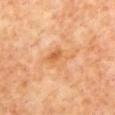<lesion>
  <biopsy_status>not biopsied; imaged during a skin examination</biopsy_status>
  <automated_metrics>
    <border_irregularity_0_10>4.0</border_irregularity_0_10>
    <color_variation_0_10>6.5</color_variation_0_10>
    <peripheral_color_asymmetry>2.0</peripheral_color_asymmetry>
    <nevus_likeness_0_100>0</nevus_likeness_0_100>
    <lesion_detection_confidence_0_100>100</lesion_detection_confidence_0_100>
  </automated_metrics>
  <image>
    <source>total-body photography crop</source>
    <field_of_view_mm>15</field_of_view_mm>
  </image>
  <lighting>cross-polarized</lighting>
  <lesion_size>
    <long_diameter_mm_approx>4.5</long_diameter_mm_approx>
  </lesion_size>
  <site>back</site>
  <patient>
    <sex>male</sex>
    <age_approx>60</age_approx>
  </patient>
</lesion>